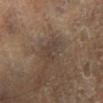Case summary:
– workup: no biopsy performed (imaged during a skin exam)
– site: the left lower leg
– automated metrics: an average lesion color of about L≈36 a*≈12 b*≈21 (CIELAB), a lesion–skin lightness drop of about 5, and a normalized border contrast of about 5; lesion-presence confidence of about 60/100
– illumination: cross-polarized illumination
– subject: male, aged around 65
– imaging modality: ~15 mm tile from a whole-body skin photo
– lesion size: ≈2.5 mm